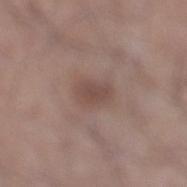Assessment: Imaged during a routine full-body skin examination; the lesion was not biopsied and no histopathology is available. Acquisition and patient details: Automated image analysis of the tile measured a mean CIELAB color near L≈47 a*≈17 b*≈22, about 8 CIELAB-L* units darker than the surrounding skin, and a normalized border contrast of about 6. It also reported a lesion-detection confidence of about 100/100. A male subject, in their 60s. A 15 mm close-up tile from a total-body photography series done for melanoma screening. The lesion is located on the leg.